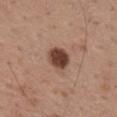notes: total-body-photography surveillance lesion; no biopsy
diameter: ~3 mm (longest diameter)
patient: male, about 55 years old
lighting: white-light
TBP lesion metrics: a footprint of about 7 mm² and a shape-asymmetry score of about 0.15 (0 = symmetric); a lesion color around L≈42 a*≈21 b*≈26 in CIELAB and roughly 17 lightness units darker than nearby skin; a border-irregularity rating of about 1.5/10, internal color variation of about 3 on a 0–10 scale, and radial color variation of about 1
body site: the mid back
imaging modality: 15 mm crop, total-body photography